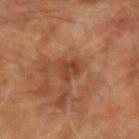The lesion was photographed on a routine skin check and not biopsied; there is no pathology result. Imaged with cross-polarized lighting. Cropped from a whole-body photographic skin survey; the tile spans about 15 mm. A male patient aged approximately 65. The recorded lesion diameter is about 3 mm. The lesion is located on the right forearm. Automated image analysis of the tile measured a mean CIELAB color near L≈41 a*≈24 b*≈32, about 8 CIELAB-L* units darker than the surrounding skin, and a normalized lesion–skin contrast near 6. And it measured a border-irregularity index near 4/10, a within-lesion color-variation index near 3/10, and a peripheral color-asymmetry measure near 1. It also reported a nevus-likeness score of about 0/100.